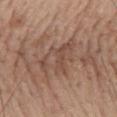Captured during whole-body skin photography for melanoma surveillance; the lesion was not biopsied. A male subject, approximately 70 years of age. A 15 mm crop from a total-body photograph taken for skin-cancer surveillance. The lesion's longest dimension is about 6 mm. On the back. An algorithmic analysis of the crop reported an eccentricity of roughly 0.8 and a shape-asymmetry score of about 0.35 (0 = symmetric). The software also gave an average lesion color of about L≈49 a*≈18 b*≈26 (CIELAB), about 7 CIELAB-L* units darker than the surrounding skin, and a normalized border contrast of about 5.5. The tile uses white-light illumination.